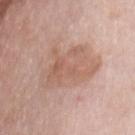The lesion was tiled from a total-body skin photograph and was not biopsied. A female subject about 75 years old. Longest diameter approximately 6 mm. On the chest. A 15 mm close-up extracted from a 3D total-body photography capture.